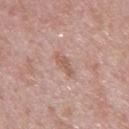<record>
<biopsy_status>not biopsied; imaged during a skin examination</biopsy_status>
<site>upper back</site>
<lighting>white-light</lighting>
<automated_metrics>
  <border_irregularity_0_10>4.5</border_irregularity_0_10>
  <color_variation_0_10>0.5</color_variation_0_10>
</automated_metrics>
<image>
  <source>total-body photography crop</source>
  <field_of_view_mm>15</field_of_view_mm>
</image>
<patient>
  <sex>male</sex>
  <age_approx>40</age_approx>
</patient>
<lesion_size>
  <long_diameter_mm_approx>3.0</long_diameter_mm_approx>
</lesion_size>
</record>